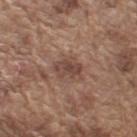{"biopsy_status": "not biopsied; imaged during a skin examination", "patient": {"sex": "male", "age_approx": 75}, "lesion_size": {"long_diameter_mm_approx": 3.5}, "automated_metrics": {"nevus_likeness_0_100": 5, "lesion_detection_confidence_0_100": 100}, "site": "right upper arm", "image": {"source": "total-body photography crop", "field_of_view_mm": 15}}